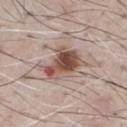Assessment:
Captured during whole-body skin photography for melanoma surveillance; the lesion was not biopsied.
Clinical summary:
A region of skin cropped from a whole-body photographic capture, roughly 15 mm wide. The tile uses white-light illumination. The patient is a male aged 53 to 57. The lesion's longest dimension is about 5 mm. The total-body-photography lesion software estimated a footprint of about 13 mm² and a symmetry-axis asymmetry near 0.35. It also reported an average lesion color of about L≈51 a*≈18 b*≈24 (CIELAB), about 15 CIELAB-L* units darker than the surrounding skin, and a lesion-to-skin contrast of about 10.5 (normalized; higher = more distinct).